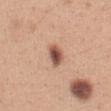The lesion was photographed on a routine skin check and not biopsied; there is no pathology result. Approximately 2.5 mm at its widest. A lesion tile, about 15 mm wide, cut from a 3D total-body photograph. A female subject, about 30 years old. Imaged with white-light lighting. On the abdomen.